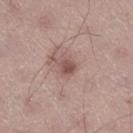notes=no biopsy performed (imaged during a skin exam); acquisition=15 mm crop, total-body photography; lighting=white-light illumination; patient=male, in their mid-40s; site=the right thigh.